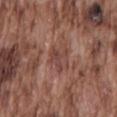The lesion was tiled from a total-body skin photograph and was not biopsied. A male subject, aged 73–77. On the mid back. A close-up tile cropped from a whole-body skin photograph, about 15 mm across.